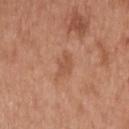biopsy status = imaged on a skin check; not biopsied
subject = male, aged 63–67
illumination = white-light
lesion diameter = ≈3 mm
anatomic site = the upper back
acquisition = ~15 mm crop, total-body skin-cancer survey